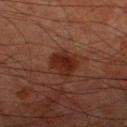Impression: The lesion was photographed on a routine skin check and not biopsied; there is no pathology result. Acquisition and patient details: A male patient approximately 80 years of age. About 3 mm across. A 15 mm crop from a total-body photograph taken for skin-cancer surveillance. Imaged with cross-polarized lighting. Automated image analysis of the tile measured a footprint of about 7 mm², an outline eccentricity of about 0.1 (0 = round, 1 = elongated), and a symmetry-axis asymmetry near 0.25. The software also gave about 7 CIELAB-L* units darker than the surrounding skin and a normalized lesion–skin contrast near 9.5. The analysis additionally found border irregularity of about 3 on a 0–10 scale and a within-lesion color-variation index near 2.5/10. The lesion is located on the leg.